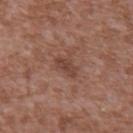Assessment:
Captured during whole-body skin photography for melanoma surveillance; the lesion was not biopsied.
Context:
Located on the back. A close-up tile cropped from a whole-body skin photograph, about 15 mm across. The patient is a male aged around 45.